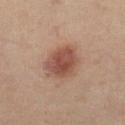notes — imaged on a skin check; not biopsied | image source — ~15 mm crop, total-body skin-cancer survey | site — the left lower leg | patient — female, aged around 40 | tile lighting — cross-polarized illumination | automated lesion analysis — a footprint of about 12 mm²; a mean CIELAB color near L≈50 a*≈22 b*≈27, about 12 CIELAB-L* units darker than the surrounding skin, and a normalized lesion–skin contrast near 8.5; a nevus-likeness score of about 100/100.